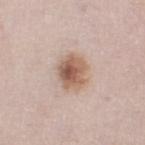Assessment: Recorded during total-body skin imaging; not selected for excision or biopsy. Acquisition and patient details: Imaged with white-light lighting. Located on the left thigh. Cropped from a total-body skin-imaging series; the visible field is about 15 mm. A male patient, aged around 80.